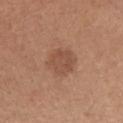biopsy_status: not biopsied; imaged during a skin examination
automated_metrics:
  eccentricity: 0.3
  shape_asymmetry: 0.15
  nevus_likeness_0_100: 25
  lesion_detection_confidence_0_100: 100
lighting: white-light
site: chest
lesion_size:
  long_diameter_mm_approx: 3.5
image:
  source: total-body photography crop
  field_of_view_mm: 15
patient:
  sex: female
  age_approx: 40